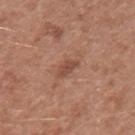The lesion was tiled from a total-body skin photograph and was not biopsied. From the left upper arm. A female subject, aged 38 to 42. This is a white-light tile. A close-up tile cropped from a whole-body skin photograph, about 15 mm across.